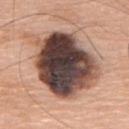This lesion was catalogued during total-body skin photography and was not selected for biopsy. A region of skin cropped from a whole-body photographic capture, roughly 15 mm wide. The subject is a male roughly 75 years of age. Located on the mid back.